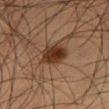notes: total-body-photography surveillance lesion; no biopsy | size: ≈3.5 mm | lighting: cross-polarized | anatomic site: the right thigh | patient: male, in their 50s | image: total-body-photography crop, ~15 mm field of view | TBP lesion metrics: a lesion color around L≈26 a*≈16 b*≈23 in CIELAB, roughly 10 lightness units darker than nearby skin, and a normalized lesion–skin contrast near 11; a border-irregularity index near 2/10.